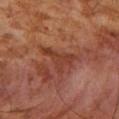This lesion was catalogued during total-body skin photography and was not selected for biopsy.
Approximately 4.5 mm at its widest.
The patient is a male aged 53–57.
The tile uses cross-polarized illumination.
A lesion tile, about 15 mm wide, cut from a 3D total-body photograph.
On the right upper arm.